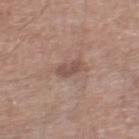Impression:
The lesion was photographed on a routine skin check and not biopsied; there is no pathology result.
Acquisition and patient details:
Longest diameter approximately 2.5 mm. This is a white-light tile. A roughly 15 mm field-of-view crop from a total-body skin photograph. A male patient, in their mid-60s. Located on the right thigh.